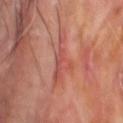biopsy status: catalogued during a skin exam; not biopsied
anatomic site: the head or neck
patient: male, aged approximately 60
image-analysis metrics: a lesion–skin lightness drop of about 7 and a normalized lesion–skin contrast near 5; a border-irregularity rating of about 7.5/10, a within-lesion color-variation index near 3.5/10, and peripheral color asymmetry of about 1
diameter: about 6 mm
image source: total-body-photography crop, ~15 mm field of view